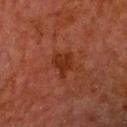Case summary:
* notes — catalogued during a skin exam; not biopsied
* automated metrics — a mean CIELAB color near L≈25 a*≈24 b*≈28 and a lesion-to-skin contrast of about 8 (normalized; higher = more distinct); border irregularity of about 4.5 on a 0–10 scale, internal color variation of about 1.5 on a 0–10 scale, and radial color variation of about 0.5
* tile lighting — cross-polarized illumination
* size — ≈3 mm
* subject — male, approximately 70 years of age
* image — ~15 mm tile from a whole-body skin photo
* body site — the head or neck